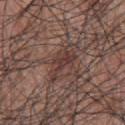Part of a total-body skin-imaging series; this lesion was reviewed on a skin check and was not flagged for biopsy. From the upper back. This image is a 15 mm lesion crop taken from a total-body photograph. The recorded lesion diameter is about 4.5 mm. The patient is a male approximately 70 years of age. Imaged with white-light lighting.